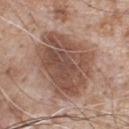{"biopsy_status": "not biopsied; imaged during a skin examination", "patient": {"sex": "male", "age_approx": 65}, "site": "chest", "image": {"source": "total-body photography crop", "field_of_view_mm": 15}, "automated_metrics": {"cielab_L": 50, "cielab_a": 19, "cielab_b": 26, "vs_skin_darker_L": 12.0, "vs_skin_contrast_norm": 9.0}, "lighting": "white-light"}